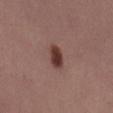Imaged during a routine full-body skin examination; the lesion was not biopsied and no histopathology is available.